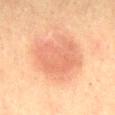A 15 mm crop from a total-body photograph taken for skin-cancer surveillance. The total-body-photography lesion software estimated a footprint of about 18 mm² and a shape eccentricity near 0.75. The analysis additionally found a lesion color around L≈56 a*≈24 b*≈31 in CIELAB, a lesion–skin lightness drop of about 8, and a lesion-to-skin contrast of about 5.5 (normalized; higher = more distinct). It also reported lesion-presence confidence of about 100/100. Located on the back. Longest diameter approximately 5.5 mm. A male subject, aged 58 to 62. Imaged with cross-polarized lighting.